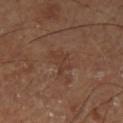biopsy_status: not biopsied; imaged during a skin examination
automated_metrics:
  area_mm2_approx: 4.5
  eccentricity: 0.65
  shape_asymmetry: 0.55
  cielab_L: 37
  cielab_a: 18
  cielab_b: 27
  vs_skin_darker_L: 5.0
  vs_skin_contrast_norm: 5.0
  border_irregularity_0_10: 6.0
  color_variation_0_10: 1.0
  peripheral_color_asymmetry: 0.5
  lesion_detection_confidence_0_100: 100
image:
  source: total-body photography crop
  field_of_view_mm: 15
site: left lower leg
lesion_size:
  long_diameter_mm_approx: 3.0
patient:
  sex: male
  age_approx: 65
lighting: cross-polarized